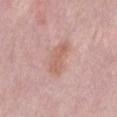| field | value |
|---|---|
| notes | no biopsy performed (imaged during a skin exam) |
| patient | male, in their mid- to late 50s |
| lighting | white-light illumination |
| anatomic site | the mid back |
| image | 15 mm crop, total-body photography |